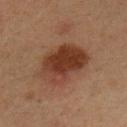{"biopsy_status": "not biopsied; imaged during a skin examination", "image": {"source": "total-body photography crop", "field_of_view_mm": 15}, "lighting": "cross-polarized", "lesion_size": {"long_diameter_mm_approx": 6.0}, "site": "chest", "patient": {"sex": "male", "age_approx": 35}}